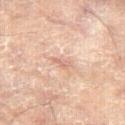{
  "biopsy_status": "not biopsied; imaged during a skin examination",
  "lighting": "cross-polarized",
  "lesion_size": {
    "long_diameter_mm_approx": 2.5
  },
  "patient": {
    "sex": "female",
    "age_approx": 80
  },
  "automated_metrics": {
    "peripheral_color_asymmetry": 0.0,
    "lesion_detection_confidence_0_100": 100
  },
  "site": "leg",
  "image": {
    "source": "total-body photography crop",
    "field_of_view_mm": 15
  }
}